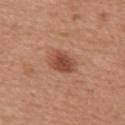follow-up: no biopsy performed (imaged during a skin exam) | imaging modality: ~15 mm crop, total-body skin-cancer survey | image-analysis metrics: an average lesion color of about L≈48 a*≈24 b*≈31 (CIELAB), about 11 CIELAB-L* units darker than the surrounding skin, and a normalized border contrast of about 8; a border-irregularity index near 2.5/10 and peripheral color asymmetry of about 1; a classifier nevus-likeness of about 90/100 and lesion-presence confidence of about 100/100 | lesion diameter: about 3.5 mm | lighting: white-light | subject: female, in their 50s | location: the arm.